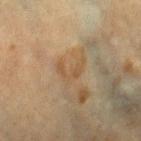Notes:
* biopsy status: total-body-photography surveillance lesion; no biopsy
* body site: the right lower leg
* patient: female, aged approximately 55
* acquisition: ~15 mm tile from a whole-body skin photo